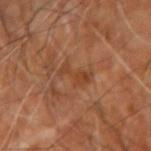biopsy status — imaged on a skin check; not biopsied
diameter — about 3.5 mm
subject — aged 63 to 67
lighting — cross-polarized
location — the left forearm
image source — total-body-photography crop, ~15 mm field of view